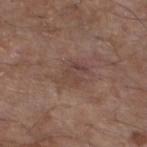No biopsy was performed on this lesion — it was imaged during a full skin examination and was not determined to be concerning. The lesion's longest dimension is about 4.5 mm. The lesion is located on the left lower leg. The patient is a male aged 78 to 82. This image is a 15 mm lesion crop taken from a total-body photograph. Imaged with white-light lighting.